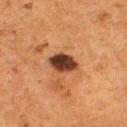Impression: This lesion was catalogued during total-body skin photography and was not selected for biopsy. Background: Automated tile analysis of the lesion measured a footprint of about 7 mm², an outline eccentricity of about 0.6 (0 = round, 1 = elongated), and two-axis asymmetry of about 0.15. A close-up tile cropped from a whole-body skin photograph, about 15 mm across. This is a cross-polarized tile. The subject is a female about 50 years old. The lesion is on the leg.